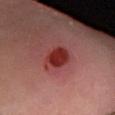Assessment: The lesion was tiled from a total-body skin photograph and was not biopsied. Image and clinical context: Automated image analysis of the tile measured a lesion area of about 8.5 mm², an outline eccentricity of about 0.7 (0 = round, 1 = elongated), and a shape-asymmetry score of about 0.25 (0 = symmetric). The analysis additionally found a nevus-likeness score of about 50/100 and a lesion-detection confidence of about 100/100. From the leg. A male patient, in their mid- to late 60s. Measured at roughly 4 mm in maximum diameter. This image is a 15 mm lesion crop taken from a total-body photograph. Captured under cross-polarized illumination.